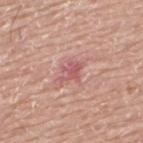Impression:
The lesion was photographed on a routine skin check and not biopsied; there is no pathology result.
Context:
A male subject, approximately 75 years of age. The lesion-visualizer software estimated a border-irregularity rating of about 5.5/10, internal color variation of about 1.5 on a 0–10 scale, and peripheral color asymmetry of about 0.5. The software also gave a classifier nevus-likeness of about 0/100 and a detector confidence of about 100 out of 100 that the crop contains a lesion. From the upper back. Cropped from a total-body skin-imaging series; the visible field is about 15 mm. The lesion's longest dimension is about 2.5 mm. This is a white-light tile.